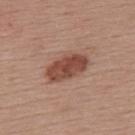Clinical impression:
This lesion was catalogued during total-body skin photography and was not selected for biopsy.
Context:
A female subject, about 55 years old. The lesion is located on the upper back. Cropped from a total-body skin-imaging series; the visible field is about 15 mm.